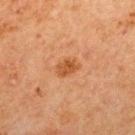Part of a total-body skin-imaging series; this lesion was reviewed on a skin check and was not flagged for biopsy.
The tile uses cross-polarized illumination.
A close-up tile cropped from a whole-body skin photograph, about 15 mm across.
The patient is a male in their mid- to late 60s.
From the mid back.